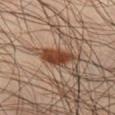Notes:
• follow-up: no biopsy performed (imaged during a skin exam)
• image: 15 mm crop, total-body photography
• patient: male, in their mid- to late 40s
• location: the left thigh